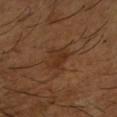This lesion was catalogued during total-body skin photography and was not selected for biopsy. A male patient aged around 65. Captured under cross-polarized illumination. From the right forearm. A region of skin cropped from a whole-body photographic capture, roughly 15 mm wide.